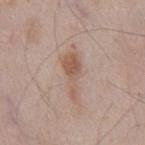A 15 mm crop from a total-body photograph taken for skin-cancer surveillance. The subject is a male in their mid- to late 50s. From the abdomen.